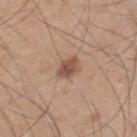Clinical impression:
Captured during whole-body skin photography for melanoma surveillance; the lesion was not biopsied.
Context:
The lesion is located on the leg. A male subject in their mid- to late 40s. A region of skin cropped from a whole-body photographic capture, roughly 15 mm wide.